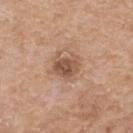Impression:
No biopsy was performed on this lesion — it was imaged during a full skin examination and was not determined to be concerning.
Clinical summary:
Automated image analysis of the tile measured an outline eccentricity of about 0.5 (0 = round, 1 = elongated). It also reported a lesion color around L≈53 a*≈20 b*≈30 in CIELAB. Longest diameter approximately 3.5 mm. The subject is a female approximately 75 years of age. The lesion is located on the upper back. A 15 mm close-up tile from a total-body photography series done for melanoma screening. The tile uses white-light illumination.